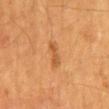Notes:
• biopsy status: total-body-photography surveillance lesion; no biopsy
• subject: male, aged 53–57
• lesion diameter: ~3.5 mm (longest diameter)
• tile lighting: cross-polarized
• TBP lesion metrics: an area of roughly 3.5 mm², an eccentricity of roughly 0.95, and a symmetry-axis asymmetry near 0.25; an average lesion color of about L≈51 a*≈24 b*≈40 (CIELAB), roughly 8 lightness units darker than nearby skin, and a normalized lesion–skin contrast near 6; border irregularity of about 3.5 on a 0–10 scale and a peripheral color-asymmetry measure near 0; a nevus-likeness score of about 35/100 and a lesion-detection confidence of about 100/100
• anatomic site: the mid back
• image source: total-body-photography crop, ~15 mm field of view